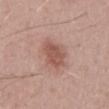{
  "biopsy_status": "not biopsied; imaged during a skin examination",
  "image": {
    "source": "total-body photography crop",
    "field_of_view_mm": 15
  },
  "patient": {
    "sex": "male",
    "age_approx": 50
  },
  "site": "mid back"
}